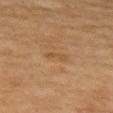The lesion was tiled from a total-body skin photograph and was not biopsied.
Located on the arm.
A female subject, aged 63–67.
Captured under cross-polarized illumination.
A 15 mm close-up tile from a total-body photography series done for melanoma screening.
Longest diameter approximately 2.5 mm.